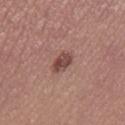Background: The lesion is located on the right thigh. A 15 mm crop from a total-body photograph taken for skin-cancer surveillance. A female subject, about 25 years old.